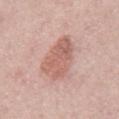Clinical impression: Captured during whole-body skin photography for melanoma surveillance; the lesion was not biopsied. Context: A 15 mm close-up tile from a total-body photography series done for melanoma screening. Captured under white-light illumination. A male subject in their mid- to late 60s. Automated image analysis of the tile measured a lesion area of about 15 mm², an eccentricity of roughly 0.9, and a symmetry-axis asymmetry near 0.25. It also reported a border-irregularity index near 3/10, a color-variation rating of about 3.5/10, and a peripheral color-asymmetry measure near 1. And it measured a detector confidence of about 100 out of 100 that the crop contains a lesion. The lesion is located on the chest.